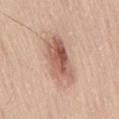workup — imaged on a skin check; not biopsied | image source — ~15 mm tile from a whole-body skin photo | patient — male, approximately 40 years of age | body site — the back | size — about 6 mm | image-analysis metrics — a lesion area of about 16 mm², a shape eccentricity near 0.85, and two-axis asymmetry of about 0.2; roughly 13 lightness units darker than nearby skin and a lesion-to-skin contrast of about 8.5 (normalized; higher = more distinct); a border-irregularity index near 2.5/10, a within-lesion color-variation index near 7.5/10, and a peripheral color-asymmetry measure near 2.5.